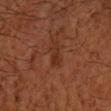biopsy_status: not biopsied; imaged during a skin examination
lesion_size:
  long_diameter_mm_approx: 2.5
site: right forearm
lighting: cross-polarized
patient:
  sex: male
  age_approx: 60
automated_metrics:
  border_irregularity_0_10: 3.5
  color_variation_0_10: 0.5
  peripheral_color_asymmetry: 0.0
  nevus_likeness_0_100: 0
  lesion_detection_confidence_0_100: 100
image:
  source: total-body photography crop
  field_of_view_mm: 15